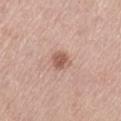| key | value |
|---|---|
| follow-up | total-body-photography surveillance lesion; no biopsy |
| patient | female, aged around 65 |
| location | the left thigh |
| TBP lesion metrics | a footprint of about 4.5 mm², an outline eccentricity of about 0.55 (0 = round, 1 = elongated), and a shape-asymmetry score of about 0.25 (0 = symmetric); a border-irregularity rating of about 2/10 and a color-variation rating of about 2.5/10 |
| size | about 2.5 mm |
| imaging modality | ~15 mm crop, total-body skin-cancer survey |